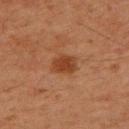Case summary:
* biopsy status · imaged on a skin check; not biopsied